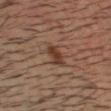biopsy status: imaged on a skin check; not biopsied
image source: ~15 mm crop, total-body skin-cancer survey
automated lesion analysis: a shape eccentricity near 0.5 and two-axis asymmetry of about 0.3; a classifier nevus-likeness of about 95/100
site: the head or neck
patient: male, aged 38–42
diameter: ~2.5 mm (longest diameter)